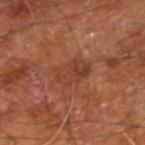Case summary:
* workup: catalogued during a skin exam; not biopsied
* acquisition: 15 mm crop, total-body photography
* anatomic site: the right leg
* subject: male, in their 60s
* lighting: cross-polarized illumination
* automated metrics: border irregularity of about 3.5 on a 0–10 scale, a color-variation rating of about 3.5/10, and radial color variation of about 1.5; a nevus-likeness score of about 15/100 and lesion-presence confidence of about 100/100
* lesion size: ~4 mm (longest diameter)